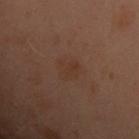Q: Was a biopsy performed?
A: total-body-photography surveillance lesion; no biopsy
Q: What are the patient's age and sex?
A: female, aged around 60
Q: What lighting was used for the tile?
A: cross-polarized illumination
Q: What is the lesion's diameter?
A: ~3 mm (longest diameter)
Q: How was this image acquired?
A: ~15 mm tile from a whole-body skin photo
Q: Where on the body is the lesion?
A: the back